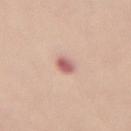The lesion was tiled from a total-body skin photograph and was not biopsied. A close-up tile cropped from a whole-body skin photograph, about 15 mm across. The lesion is located on the lower back. The subject is a female aged 48 to 52.